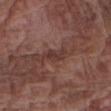| feature | finding |
|---|---|
| workup | imaged on a skin check; not biopsied |
| location | the right upper arm |
| diameter | ≈2.5 mm |
| acquisition | ~15 mm crop, total-body skin-cancer survey |
| subject | male, aged approximately 75 |